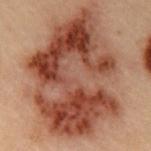No biopsy was performed on this lesion — it was imaged during a full skin examination and was not determined to be concerning. The patient is a male aged around 55. Imaged with cross-polarized lighting. Approximately 14 mm at its widest. A 15 mm crop from a total-body photograph taken for skin-cancer surveillance. An algorithmic analysis of the crop reported an area of roughly 95 mm² and a symmetry-axis asymmetry near 0.2. The software also gave a classifier nevus-likeness of about 0/100 and a detector confidence of about 100 out of 100 that the crop contains a lesion. The lesion is located on the chest.